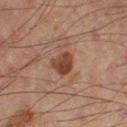Clinical impression: This lesion was catalogued during total-body skin photography and was not selected for biopsy. Image and clinical context: The lesion's longest dimension is about 2.5 mm. On the leg. The total-body-photography lesion software estimated a footprint of about 5.5 mm², an eccentricity of roughly 0.4, and two-axis asymmetry of about 0.3. The analysis additionally found a mean CIELAB color near L≈31 a*≈18 b*≈22, a lesion–skin lightness drop of about 9, and a normalized lesion–skin contrast near 9. It also reported a border-irregularity rating of about 2.5/10, internal color variation of about 4 on a 0–10 scale, and peripheral color asymmetry of about 1.5. It also reported a nevus-likeness score of about 80/100 and a detector confidence of about 100 out of 100 that the crop contains a lesion. A male patient roughly 60 years of age. The tile uses cross-polarized illumination. A 15 mm close-up extracted from a 3D total-body photography capture.